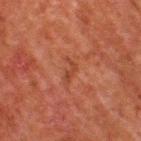Notes:
- biopsy status: catalogued during a skin exam; not biopsied
- automated metrics: a lesion color around L≈33 a*≈23 b*≈27 in CIELAB, about 5 CIELAB-L* units darker than the surrounding skin, and a lesion-to-skin contrast of about 5.5 (normalized; higher = more distinct); a border-irregularity index near 7/10 and a color-variation rating of about 0/10
- diameter: ~3 mm (longest diameter)
- anatomic site: the upper back
- patient: male, aged 58 to 62
- tile lighting: cross-polarized illumination
- imaging modality: ~15 mm crop, total-body skin-cancer survey